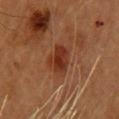follow-up: no biopsy performed (imaged during a skin exam)
imaging modality: ~15 mm tile from a whole-body skin photo
subject: female, approximately 60 years of age
body site: the upper back